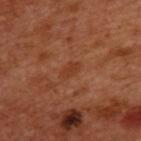Q: Was this lesion biopsied?
A: imaged on a skin check; not biopsied
Q: Where on the body is the lesion?
A: the upper back
Q: What kind of image is this?
A: 15 mm crop, total-body photography
Q: Patient demographics?
A: male, approximately 50 years of age